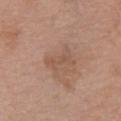Q: How was this image acquired?
A: ~15 mm crop, total-body skin-cancer survey
Q: How was the tile lit?
A: white-light
Q: What are the patient's age and sex?
A: female, aged around 60
Q: What is the lesion's diameter?
A: ~3.5 mm (longest diameter)
Q: Where on the body is the lesion?
A: the chest